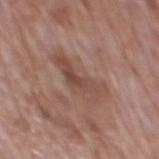<lesion>
<biopsy_status>not biopsied; imaged during a skin examination</biopsy_status>
<automated_metrics>
  <eccentricity>0.9</eccentricity>
  <shape_asymmetry>0.4</shape_asymmetry>
  <vs_skin_darker_L>8.0</vs_skin_darker_L>
  <nevus_likeness_0_100>0</nevus_likeness_0_100>
  <lesion_detection_confidence_0_100>95</lesion_detection_confidence_0_100>
</automated_metrics>
<image>
  <source>total-body photography crop</source>
  <field_of_view_mm>15</field_of_view_mm>
</image>
<site>mid back</site>
<lesion_size>
  <long_diameter_mm_approx>6.0</long_diameter_mm_approx>
</lesion_size>
<patient>
  <sex>male</sex>
  <age_approx>70</age_approx>
</patient>
</lesion>